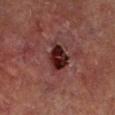The lesion was tiled from a total-body skin photograph and was not biopsied. A roughly 15 mm field-of-view crop from a total-body skin photograph. The lesion-visualizer software estimated an area of roughly 7 mm², a shape eccentricity near 0.7, and a symmetry-axis asymmetry near 0.25. The analysis additionally found a lesion–skin lightness drop of about 13. And it measured internal color variation of about 5.5 on a 0–10 scale. About 3.5 mm across. The patient is a male aged 68–72. The lesion is located on the left lower leg. This is a cross-polarized tile.